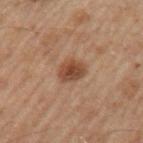{"biopsy_status": "not biopsied; imaged during a skin examination", "image": {"source": "total-body photography crop", "field_of_view_mm": 15}, "patient": {"sex": "male", "age_approx": 60}, "site": "left upper arm"}